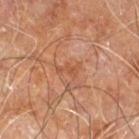Imaged during a routine full-body skin examination; the lesion was not biopsied and no histopathology is available. This is a cross-polarized tile. From the right leg. The patient is a male roughly 60 years of age. The lesion's longest dimension is about 2.5 mm. A 15 mm close-up extracted from a 3D total-body photography capture. The total-body-photography lesion software estimated a lesion color around L≈50 a*≈25 b*≈34 in CIELAB, a lesion–skin lightness drop of about 6, and a lesion-to-skin contrast of about 5 (normalized; higher = more distinct). The software also gave a border-irregularity index near 6/10.